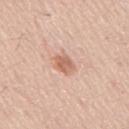Assessment: Recorded during total-body skin imaging; not selected for excision or biopsy. Acquisition and patient details: The subject is a male in their 60s. A roughly 15 mm field-of-view crop from a total-body skin photograph. The lesion-visualizer software estimated an automated nevus-likeness rating near 55 out of 100. On the mid back.